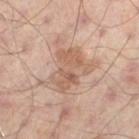Q: Was a biopsy performed?
A: imaged on a skin check; not biopsied
Q: Automated lesion metrics?
A: a mean CIELAB color near L≈56 a*≈18 b*≈28, roughly 8 lightness units darker than nearby skin, and a normalized border contrast of about 6
Q: What is the anatomic site?
A: the leg
Q: What is the imaging modality?
A: total-body-photography crop, ~15 mm field of view
Q: What lighting was used for the tile?
A: cross-polarized illumination
Q: Patient demographics?
A: male, roughly 65 years of age